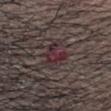biopsy_status: not biopsied; imaged during a skin examination
lesion_size:
  long_diameter_mm_approx: 2.5
image:
  source: total-body photography crop
  field_of_view_mm: 15
site: head or neck
patient:
  sex: male
  age_approx: 40